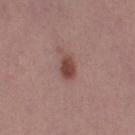| feature | finding |
|---|---|
| follow-up | catalogued during a skin exam; not biopsied |
| image | ~15 mm tile from a whole-body skin photo |
| anatomic site | the right thigh |
| size | ≈3 mm |
| lighting | white-light illumination |
| image-analysis metrics | a within-lesion color-variation index near 2.5/10 and peripheral color asymmetry of about 1 |
| subject | female, aged approximately 50 |